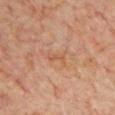Findings:
– follow-up — imaged on a skin check; not biopsied
– imaging modality — total-body-photography crop, ~15 mm field of view
– subject — male, in their 70s
– site — the front of the torso